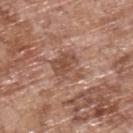follow-up: imaged on a skin check; not biopsied
tile lighting: white-light
site: the back
acquisition: ~15 mm tile from a whole-body skin photo
patient: male, aged approximately 70